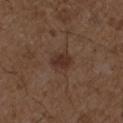Captured during whole-body skin photography for melanoma surveillance; the lesion was not biopsied.